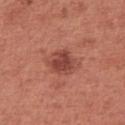This lesion was catalogued during total-body skin photography and was not selected for biopsy.
On the right upper arm.
A female subject, aged approximately 50.
A lesion tile, about 15 mm wide, cut from a 3D total-body photograph.